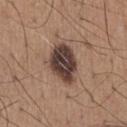Imaged during a routine full-body skin examination; the lesion was not biopsied and no histopathology is available.
The subject is a male roughly 65 years of age.
A 15 mm crop from a total-body photograph taken for skin-cancer surveillance.
The lesion is on the chest.